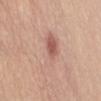Recorded during total-body skin imaging; not selected for excision or biopsy. The subject is a female aged around 75. The lesion is located on the abdomen. A lesion tile, about 15 mm wide, cut from a 3D total-body photograph.